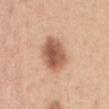Q: Is there a histopathology result?
A: catalogued during a skin exam; not biopsied
Q: What is the imaging modality?
A: ~15 mm crop, total-body skin-cancer survey
Q: Automated lesion metrics?
A: an average lesion color of about L≈57 a*≈23 b*≈31 (CIELAB), roughly 15 lightness units darker than nearby skin, and a lesion-to-skin contrast of about 9.5 (normalized; higher = more distinct); a border-irregularity rating of about 1.5/10, a color-variation rating of about 5/10, and radial color variation of about 1.5
Q: Who is the patient?
A: female, in their 30s
Q: What is the anatomic site?
A: the chest